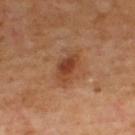Part of a total-body skin-imaging series; this lesion was reviewed on a skin check and was not flagged for biopsy. Imaged with cross-polarized lighting. Cropped from a total-body skin-imaging series; the visible field is about 15 mm. On the upper back. A male subject aged around 70. The recorded lesion diameter is about 4 mm.